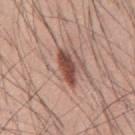Q: Was a biopsy performed?
A: no biopsy performed (imaged during a skin exam)
Q: What is the imaging modality?
A: 15 mm crop, total-body photography
Q: Patient demographics?
A: male, approximately 60 years of age
Q: Illumination type?
A: white-light
Q: Lesion location?
A: the mid back